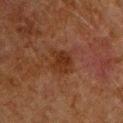Findings:
• acquisition · ~15 mm crop, total-body skin-cancer survey
• anatomic site · the front of the torso
• subject · male, about 60 years old